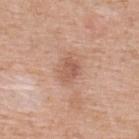• workup · no biopsy performed (imaged during a skin exam)
• patient · female, approximately 40 years of age
• tile lighting · white-light
• automated lesion analysis · a footprint of about 6 mm², an outline eccentricity of about 0.75 (0 = round, 1 = elongated), and a symmetry-axis asymmetry near 0.25; a mean CIELAB color near L≈57 a*≈22 b*≈29, roughly 9 lightness units darker than nearby skin, and a lesion-to-skin contrast of about 6 (normalized; higher = more distinct); a color-variation rating of about 3/10 and radial color variation of about 1; a lesion-detection confidence of about 100/100
• lesion diameter · about 3.5 mm
• image · ~15 mm crop, total-body skin-cancer survey
• body site · the upper back